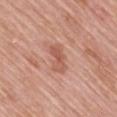{"biopsy_status": "not biopsied; imaged during a skin examination", "site": "mid back", "lighting": "white-light", "image": {"source": "total-body photography crop", "field_of_view_mm": 15}, "lesion_size": {"long_diameter_mm_approx": 3.0}, "automated_metrics": {"cielab_L": 57, "cielab_a": 25, "cielab_b": 30, "vs_skin_darker_L": 9.0}, "patient": {"sex": "female", "age_approx": 60}}